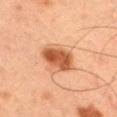<lesion>
  <biopsy_status>not biopsied; imaged during a skin examination</biopsy_status>
  <patient>
    <sex>male</sex>
    <age_approx>50</age_approx>
  </patient>
  <site>right thigh</site>
  <image>
    <source>total-body photography crop</source>
    <field_of_view_mm>15</field_of_view_mm>
  </image>
</lesion>